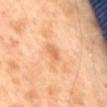notes = total-body-photography surveillance lesion; no biopsy
acquisition = total-body-photography crop, ~15 mm field of view
site = the abdomen
patient = male, aged approximately 60
illumination = cross-polarized